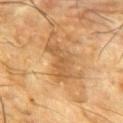Q: Was a biopsy performed?
A: no biopsy performed (imaged during a skin exam)
Q: Lesion location?
A: the chest
Q: Lesion size?
A: ~5 mm (longest diameter)
Q: What did automated image analysis measure?
A: a border-irregularity rating of about 5.5/10 and a peripheral color-asymmetry measure near 1
Q: What lighting was used for the tile?
A: cross-polarized illumination
Q: What is the imaging modality?
A: ~15 mm tile from a whole-body skin photo
Q: Who is the patient?
A: male, aged around 85 or older